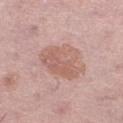Notes:
* follow-up: total-body-photography surveillance lesion; no biopsy
* patient: female, aged 48 to 52
* automated metrics: a footprint of about 17 mm², an outline eccentricity of about 0.65 (0 = round, 1 = elongated), and a symmetry-axis asymmetry near 0.3; roughly 8 lightness units darker than nearby skin; a nevus-likeness score of about 40/100
* image: ~15 mm crop, total-body skin-cancer survey
* lighting: white-light
* body site: the left lower leg